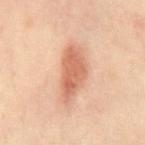Findings:
- biopsy status · imaged on a skin check; not biopsied
- location · the mid back
- acquisition · ~15 mm tile from a whole-body skin photo
- lesion size · about 6 mm
- subject · male, approximately 45 years of age